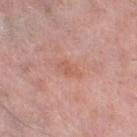Acquisition and patient details:
A male patient in their mid-50s. The lesion is located on the right lower leg. A region of skin cropped from a whole-body photographic capture, roughly 15 mm wide.